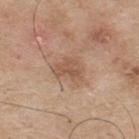follow-up: total-body-photography surveillance lesion; no biopsy | TBP lesion metrics: a symmetry-axis asymmetry near 0.4; a border-irregularity rating of about 4/10, a within-lesion color-variation index near 2/10, and a peripheral color-asymmetry measure near 0.5 | patient: male, aged 73–77 | lighting: white-light illumination | diameter: about 3.5 mm | acquisition: ~15 mm crop, total-body skin-cancer survey | body site: the upper back.